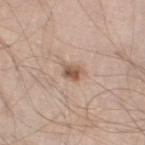Impression: Imaged during a routine full-body skin examination; the lesion was not biopsied and no histopathology is available. Clinical summary: Located on the leg. Measured at roughly 2.5 mm in maximum diameter. Automated tile analysis of the lesion measured a lesion color around L≈56 a*≈18 b*≈28 in CIELAB, a lesion–skin lightness drop of about 12, and a normalized lesion–skin contrast near 8. And it measured a border-irregularity index near 2.5/10, a within-lesion color-variation index near 3/10, and radial color variation of about 1. The software also gave a lesion-detection confidence of about 100/100. The subject is a male aged 58 to 62. The tile uses white-light illumination. A roughly 15 mm field-of-view crop from a total-body skin photograph.